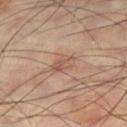Recorded during total-body skin imaging; not selected for excision or biopsy.
This is a cross-polarized tile.
The subject is a male roughly 60 years of age.
The recorded lesion diameter is about 3.5 mm.
The lesion is located on the right thigh.
Cropped from a whole-body photographic skin survey; the tile spans about 15 mm.